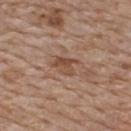The lesion was photographed on a routine skin check and not biopsied; there is no pathology result. A male subject about 80 years old. A 15 mm crop from a total-body photograph taken for skin-cancer surveillance. From the back. The recorded lesion diameter is about 3 mm. The tile uses white-light illumination.